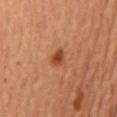Impression: Part of a total-body skin-imaging series; this lesion was reviewed on a skin check and was not flagged for biopsy. Context: On the front of the torso. This image is a 15 mm lesion crop taken from a total-body photograph. A male subject, aged 63–67.